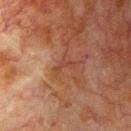notes = no biopsy performed (imaged during a skin exam) | acquisition = 15 mm crop, total-body photography | TBP lesion metrics = a mean CIELAB color near L≈34 a*≈22 b*≈25, roughly 5 lightness units darker than nearby skin, and a normalized lesion–skin contrast near 5; border irregularity of about 7 on a 0–10 scale, internal color variation of about 0 on a 0–10 scale, and peripheral color asymmetry of about 0; a nevus-likeness score of about 0/100 and lesion-presence confidence of about 60/100 | size = ~3 mm (longest diameter) | patient = male, aged 78 to 82 | body site = the chest.